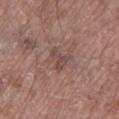workup: imaged on a skin check; not biopsied | automated lesion analysis: an outline eccentricity of about 0.75 (0 = round, 1 = elongated) and a symmetry-axis asymmetry near 0.55 | patient: male, approximately 80 years of age | lesion size: about 3 mm | body site: the left lower leg | imaging modality: 15 mm crop, total-body photography.